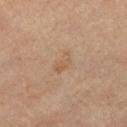biopsy_status: not biopsied; imaged during a skin examination
image:
  source: total-body photography crop
  field_of_view_mm: 15
site: left lower leg
patient:
  sex: female
  age_approx: 65
automated_metrics:
  area_mm2_approx: 4.0
  eccentricity: 0.8
  lesion_detection_confidence_0_100: 100
lesion_size:
  long_diameter_mm_approx: 3.0
lighting: cross-polarized